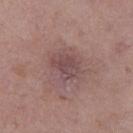Captured during whole-body skin photography for melanoma surveillance; the lesion was not biopsied.
The subject is a male about 70 years old.
Captured under white-light illumination.
A 15 mm close-up extracted from a 3D total-body photography capture.
Longest diameter approximately 3.5 mm.
The lesion is on the left thigh.